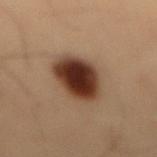Findings:
- lighting: cross-polarized illumination
- image: ~15 mm crop, total-body skin-cancer survey
- anatomic site: the lower back
- patient: male, aged around 55